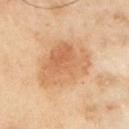follow-up: catalogued during a skin exam; not biopsied
subject: male, roughly 55 years of age
anatomic site: the left upper arm
lesion size: ~7.5 mm (longest diameter)
TBP lesion metrics: a lesion area of about 26 mm²; an average lesion color of about L≈51 a*≈17 b*≈32 (CIELAB), about 8 CIELAB-L* units darker than the surrounding skin, and a normalized lesion–skin contrast near 6.5
image source: ~15 mm crop, total-body skin-cancer survey
tile lighting: cross-polarized illumination